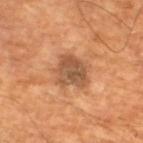  biopsy_status: not biopsied; imaged during a skin examination
  site: leg
  image:
    source: total-body photography crop
    field_of_view_mm: 15
  patient:
    sex: male
    age_approx: 65